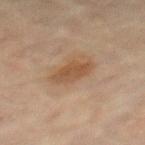Case summary:
• biopsy status — no biopsy performed (imaged during a skin exam)
• lesion size — ~4 mm (longest diameter)
• image — ~15 mm crop, total-body skin-cancer survey
• subject — female, approximately 80 years of age
• tile lighting — cross-polarized
• anatomic site — the left thigh